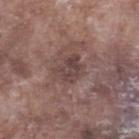<tbp_lesion>
  <biopsy_status>not biopsied; imaged during a skin examination</biopsy_status>
  <lighting>white-light</lighting>
  <patient>
    <sex>male</sex>
    <age_approx>75</age_approx>
  </patient>
  <image>
    <source>total-body photography crop</source>
    <field_of_view_mm>15</field_of_view_mm>
  </image>
  <site>right lower leg</site>
  <lesion_size>
    <long_diameter_mm_approx>3.0</long_diameter_mm_approx>
  </lesion_size>
</tbp_lesion>